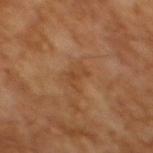• follow-up: no biopsy performed (imaged during a skin exam)
• image source: ~15 mm tile from a whole-body skin photo
• patient: male, aged approximately 65